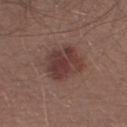Clinical impression:
Captured during whole-body skin photography for melanoma surveillance; the lesion was not biopsied.
Context:
The lesion is located on the arm. The patient is a male about 55 years old. A 15 mm close-up extracted from a 3D total-body photography capture. Imaged with white-light lighting. About 4.5 mm across.